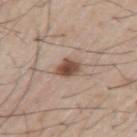biopsy_status: not biopsied; imaged during a skin examination
lighting: white-light
automated_metrics:
  cielab_L: 50
  cielab_a: 18
  cielab_b: 26
  vs_skin_darker_L: 14.0
  vs_skin_contrast_norm: 10.0
patient:
  sex: male
  age_approx: 55
lesion_size:
  long_diameter_mm_approx: 2.5
image:
  source: total-body photography crop
  field_of_view_mm: 15